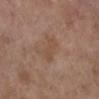Impression:
The lesion was tiled from a total-body skin photograph and was not biopsied.
Acquisition and patient details:
A female subject approximately 65 years of age. The lesion is on the right lower leg. A 15 mm close-up extracted from a 3D total-body photography capture. Measured at roughly 3.5 mm in maximum diameter. This is a white-light tile.